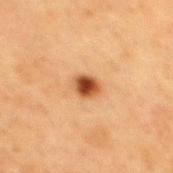notes: no biopsy performed (imaged during a skin exam)
image: total-body-photography crop, ~15 mm field of view
automated metrics: an average lesion color of about L≈44 a*≈24 b*≈36 (CIELAB), a lesion–skin lightness drop of about 17, and a lesion-to-skin contrast of about 12 (normalized; higher = more distinct); a border-irregularity index near 2/10 and a color-variation rating of about 5/10; an automated nevus-likeness rating near 100 out of 100
site: the mid back
illumination: cross-polarized illumination
subject: female, about 40 years old